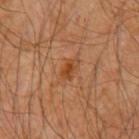biopsy status: imaged on a skin check; not biopsied | site: the left upper arm | illumination: cross-polarized illumination | subject: male, aged around 60 | image: ~15 mm crop, total-body skin-cancer survey.